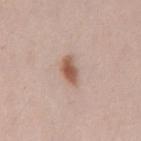Case summary:
• automated metrics · border irregularity of about 2 on a 0–10 scale, a within-lesion color-variation index near 3.5/10, and a peripheral color-asymmetry measure near 1; a nevus-likeness score of about 100/100 and lesion-presence confidence of about 100/100
• illumination · white-light illumination
• lesion diameter · ~3.5 mm (longest diameter)
• site · the back
• subject · male, in their mid-30s
• imaging modality · total-body-photography crop, ~15 mm field of view